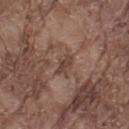Captured during whole-body skin photography for melanoma surveillance; the lesion was not biopsied.
Automated tile analysis of the lesion measured an area of roughly 3 mm², an outline eccentricity of about 0.9 (0 = round, 1 = elongated), and two-axis asymmetry of about 0.35. And it measured a border-irregularity index near 4/10, internal color variation of about 0 on a 0–10 scale, and radial color variation of about 0. The software also gave a nevus-likeness score of about 0/100 and a lesion-detection confidence of about 50/100.
From the left thigh.
A lesion tile, about 15 mm wide, cut from a 3D total-body photograph.
A male patient, aged around 80.